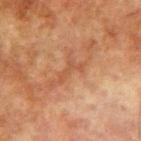Assessment:
Captured during whole-body skin photography for melanoma surveillance; the lesion was not biopsied.
Clinical summary:
This image is a 15 mm lesion crop taken from a total-body photograph. Located on the right upper arm. A male subject aged 73 to 77. Automated image analysis of the tile measured a border-irregularity index near 8/10, a within-lesion color-variation index near 0/10, and radial color variation of about 0. And it measured a nevus-likeness score of about 0/100 and a lesion-detection confidence of about 90/100. Approximately 3 mm at its widest.